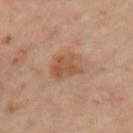Findings:
- workup · no biopsy performed (imaged during a skin exam)
- illumination · cross-polarized illumination
- lesion size · ~4 mm (longest diameter)
- body site · the left upper arm
- image source · ~15 mm tile from a whole-body skin photo
- TBP lesion metrics · a detector confidence of about 100 out of 100 that the crop contains a lesion
- subject · female, aged 43–47